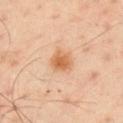Clinical impression: No biopsy was performed on this lesion — it was imaged during a full skin examination and was not determined to be concerning. Context: From the left arm. A male patient, aged 48–52. Cropped from a whole-body photographic skin survey; the tile spans about 15 mm. The tile uses cross-polarized illumination. Measured at roughly 3 mm in maximum diameter.